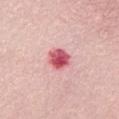Q: How was this image acquired?
A: ~15 mm crop, total-body skin-cancer survey
Q: Who is the patient?
A: female, aged around 55
Q: Where on the body is the lesion?
A: the chest